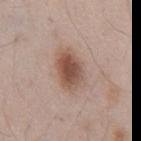The lesion was tiled from a total-body skin photograph and was not biopsied.
The lesion is on the abdomen.
Approximately 5 mm at its widest.
A male subject approximately 55 years of age.
A close-up tile cropped from a whole-body skin photograph, about 15 mm across.
Captured under white-light illumination.
The total-body-photography lesion software estimated a lesion area of about 11 mm², an eccentricity of roughly 0.75, and a shape-asymmetry score of about 0.2 (0 = symmetric). The software also gave a classifier nevus-likeness of about 95/100 and lesion-presence confidence of about 100/100.